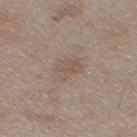biopsy_status: not biopsied; imaged during a skin examination
site: right thigh
patient:
  sex: male
  age_approx: 50
automated_metrics:
  area_mm2_approx: 5.5
  vs_skin_darker_L: 6.0
  vs_skin_contrast_norm: 4.5
  nevus_likeness_0_100: 0
  lesion_detection_confidence_0_100: 100
lesion_size:
  long_diameter_mm_approx: 3.0
lighting: white-light
image:
  source: total-body photography crop
  field_of_view_mm: 15